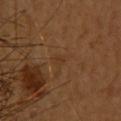Recorded during total-body skin imaging; not selected for excision or biopsy. The total-body-photography lesion software estimated a mean CIELAB color near L≈34 a*≈19 b*≈31, a lesion–skin lightness drop of about 4, and a lesion-to-skin contrast of about 4.5 (normalized; higher = more distinct). A female subject, in their mid- to late 50s. About 1.5 mm across. A 15 mm crop from a total-body photograph taken for skin-cancer surveillance. This is a cross-polarized tile. Located on the upper back.